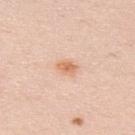The lesion was tiled from a total-body skin photograph and was not biopsied.
This image is a 15 mm lesion crop taken from a total-body photograph.
Imaged with white-light lighting.
Measured at roughly 2.5 mm in maximum diameter.
The lesion-visualizer software estimated a mean CIELAB color near L≈69 a*≈22 b*≈36.
A male patient aged around 35.
From the upper back.